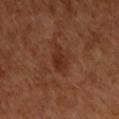Imaged during a routine full-body skin examination; the lesion was not biopsied and no histopathology is available.
Automated tile analysis of the lesion measured a lesion area of about 6 mm², an eccentricity of roughly 0.8, and a symmetry-axis asymmetry near 0.3. The analysis additionally found internal color variation of about 2.5 on a 0–10 scale and a peripheral color-asymmetry measure near 0.5. It also reported a classifier nevus-likeness of about 5/100 and a detector confidence of about 100 out of 100 that the crop contains a lesion.
From the chest.
A male subject aged 48–52.
A 15 mm close-up tile from a total-body photography series done for melanoma screening.
Captured under cross-polarized illumination.